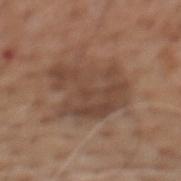{"biopsy_status": "not biopsied; imaged during a skin examination", "automated_metrics": {"area_mm2_approx": 24.0, "eccentricity": 0.75, "shape_asymmetry": 0.4, "cielab_L": 44, "cielab_a": 17, "cielab_b": 27, "vs_skin_contrast_norm": 7.0, "border_irregularity_0_10": 5.0, "color_variation_0_10": 3.5, "peripheral_color_asymmetry": 1.0}, "site": "back", "image": {"source": "total-body photography crop", "field_of_view_mm": 15}, "lighting": "white-light", "lesion_size": {"long_diameter_mm_approx": 7.0}, "patient": {"sex": "male", "age_approx": 75}}